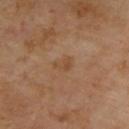Q: Is there a histopathology result?
A: catalogued during a skin exam; not biopsied
Q: Lesion size?
A: about 2.5 mm
Q: How was this image acquired?
A: total-body-photography crop, ~15 mm field of view
Q: Patient demographics?
A: male, aged 68 to 72
Q: Illumination type?
A: cross-polarized illumination
Q: What did automated image analysis measure?
A: an average lesion color of about L≈46 a*≈18 b*≈32 (CIELAB) and about 5 CIELAB-L* units darker than the surrounding skin; border irregularity of about 2.5 on a 0–10 scale, internal color variation of about 2 on a 0–10 scale, and radial color variation of about 0.5
Q: What is the anatomic site?
A: the upper back